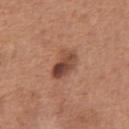Clinical impression: Part of a total-body skin-imaging series; this lesion was reviewed on a skin check and was not flagged for biopsy. Context: The patient is a male aged approximately 65. A roughly 15 mm field-of-view crop from a total-body skin photograph. Captured under white-light illumination. The lesion's longest dimension is about 4 mm. Located on the front of the torso.